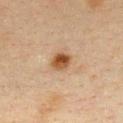Q: What did automated image analysis measure?
A: a shape eccentricity near 0.55
Q: How was this image acquired?
A: total-body-photography crop, ~15 mm field of view
Q: What lighting was used for the tile?
A: cross-polarized illumination
Q: What is the lesion's diameter?
A: about 2.5 mm
Q: What are the patient's age and sex?
A: female, aged 38–42
Q: What is the anatomic site?
A: the chest